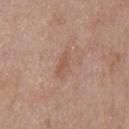{
  "biopsy_status": "not biopsied; imaged during a skin examination",
  "lesion_size": {
    "long_diameter_mm_approx": 4.0
  },
  "patient": {
    "sex": "male",
    "age_approx": 55
  },
  "site": "chest",
  "image": {
    "source": "total-body photography crop",
    "field_of_view_mm": 15
  },
  "lighting": "white-light"
}